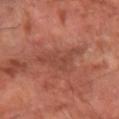Clinical impression:
The lesion was photographed on a routine skin check and not biopsied; there is no pathology result.
Acquisition and patient details:
The subject is approximately 65 years of age. On the left forearm. A region of skin cropped from a whole-body photographic capture, roughly 15 mm wide. Imaged with cross-polarized lighting. The recorded lesion diameter is about 5.5 mm. Automated tile analysis of the lesion measured a border-irregularity index near 8/10, internal color variation of about 2 on a 0–10 scale, and peripheral color asymmetry of about 0.5. The analysis additionally found a classifier nevus-likeness of about 0/100 and a lesion-detection confidence of about 70/100.